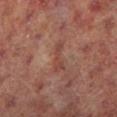{
  "site": "left lower leg",
  "patient": {
    "sex": "male",
    "age_approx": 65
  },
  "image": {
    "source": "total-body photography crop",
    "field_of_view_mm": 15
  },
  "lighting": "cross-polarized",
  "lesion_size": {
    "long_diameter_mm_approx": 3.5
  }
}